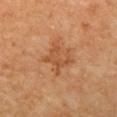{"image": {"source": "total-body photography crop", "field_of_view_mm": 15}, "site": "upper back", "lighting": "cross-polarized", "patient": {"sex": "female"}, "automated_metrics": {"cielab_L": 54, "cielab_a": 26, "cielab_b": 40, "vs_skin_darker_L": 8.0, "border_irregularity_0_10": 4.5, "color_variation_0_10": 3.0, "nevus_likeness_0_100": 5, "lesion_detection_confidence_0_100": 100}, "lesion_size": {"long_diameter_mm_approx": 3.5}}